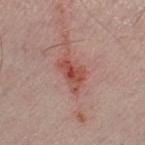Part of a total-body skin-imaging series; this lesion was reviewed on a skin check and was not flagged for biopsy. About 4.5 mm across. The lesion is on the right lower leg. The patient is a male aged around 60. A 15 mm crop from a total-body photograph taken for skin-cancer surveillance. Imaged with white-light lighting.